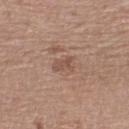Q: Was a biopsy performed?
A: imaged on a skin check; not biopsied
Q: Lesion size?
A: about 2.5 mm
Q: How was this image acquired?
A: ~15 mm crop, total-body skin-cancer survey
Q: Where on the body is the lesion?
A: the right lower leg
Q: Patient demographics?
A: female, about 55 years old